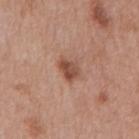Q: Was this lesion biopsied?
A: imaged on a skin check; not biopsied
Q: Automated lesion metrics?
A: an average lesion color of about L≈49 a*≈22 b*≈29 (CIELAB), roughly 12 lightness units darker than nearby skin, and a lesion-to-skin contrast of about 8.5 (normalized; higher = more distinct); a border-irregularity index near 2.5/10, a within-lesion color-variation index near 3.5/10, and radial color variation of about 1.5; a classifier nevus-likeness of about 65/100 and a lesion-detection confidence of about 100/100
Q: What is the imaging modality?
A: total-body-photography crop, ~15 mm field of view
Q: Where on the body is the lesion?
A: the mid back
Q: Who is the patient?
A: male, approximately 70 years of age
Q: What is the lesion's diameter?
A: ~3 mm (longest diameter)